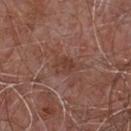Findings:
- notes · total-body-photography surveillance lesion; no biopsy
- lesion size · ≈2.5 mm
- subject · male, in their mid- to late 50s
- imaging modality · ~15 mm crop, total-body skin-cancer survey
- location · the front of the torso